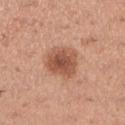{"biopsy_status": "not biopsied; imaged during a skin examination", "lighting": "white-light", "image": {"source": "total-body photography crop", "field_of_view_mm": 15}, "site": "left lower leg", "patient": {"sex": "female", "age_approx": 30}, "lesion_size": {"long_diameter_mm_approx": 4.5}}